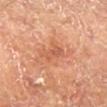follow-up: imaged on a skin check; not biopsied
acquisition: total-body-photography crop, ~15 mm field of view
lesion diameter: about 3.5 mm
body site: the right lower leg
patient: male, roughly 70 years of age
illumination: cross-polarized